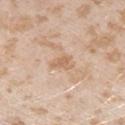Captured during whole-body skin photography for melanoma surveillance; the lesion was not biopsied.
A female subject, aged 23 to 27.
The lesion is located on the left upper arm.
A 15 mm crop from a total-body photograph taken for skin-cancer surveillance.
This is a white-light tile.
Automated image analysis of the tile measured a lesion area of about 3.5 mm², an outline eccentricity of about 0.75 (0 = round, 1 = elongated), and a symmetry-axis asymmetry near 0.45. It also reported internal color variation of about 1.5 on a 0–10 scale and a peripheral color-asymmetry measure near 0.5. It also reported an automated nevus-likeness rating near 0 out of 100.
The lesion's longest dimension is about 2.5 mm.